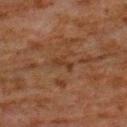Impression: Captured during whole-body skin photography for melanoma surveillance; the lesion was not biopsied. Clinical summary: This is a cross-polarized tile. Measured at roughly 2.5 mm in maximum diameter. The lesion is located on the left upper arm. Automated image analysis of the tile measured a lesion color around L≈28 a*≈17 b*≈26 in CIELAB, roughly 5 lightness units darker than nearby skin, and a normalized border contrast of about 6. And it measured a border-irregularity index near 4/10 and a peripheral color-asymmetry measure near 0. A region of skin cropped from a whole-body photographic capture, roughly 15 mm wide. A male patient, in their 80s.